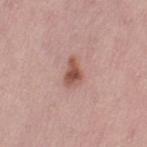biopsy status = catalogued during a skin exam; not biopsied
imaging modality = 15 mm crop, total-body photography
lighting = white-light illumination
subject = female, roughly 50 years of age
anatomic site = the leg